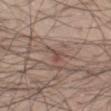Assessment:
Imaged during a routine full-body skin examination; the lesion was not biopsied and no histopathology is available.
Context:
Cropped from a whole-body photographic skin survey; the tile spans about 15 mm. From the left thigh. A male subject in their mid-50s.